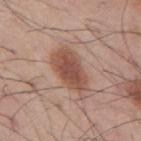Q: Was a biopsy performed?
A: no biopsy performed (imaged during a skin exam)
Q: What is the imaging modality?
A: total-body-photography crop, ~15 mm field of view
Q: How was the tile lit?
A: white-light illumination
Q: Patient demographics?
A: male, aged 53–57
Q: Lesion size?
A: ~6 mm (longest diameter)
Q: Where on the body is the lesion?
A: the mid back